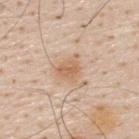Case summary:
– workup: imaged on a skin check; not biopsied
– subject: male, in their 60s
– image: 15 mm crop, total-body photography
– site: the upper back